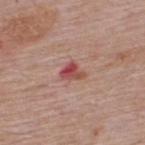Impression:
Recorded during total-body skin imaging; not selected for excision or biopsy.
Context:
Captured under white-light illumination. The total-body-photography lesion software estimated border irregularity of about 4 on a 0–10 scale and a color-variation rating of about 6.5/10. And it measured an automated nevus-likeness rating near 0 out of 100. A male subject, aged approximately 65. Located on the upper back. Approximately 2.5 mm at its widest. A 15 mm close-up extracted from a 3D total-body photography capture.